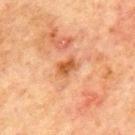Recorded during total-body skin imaging; not selected for excision or biopsy.
On the mid back.
Cropped from a total-body skin-imaging series; the visible field is about 15 mm.
This is a cross-polarized tile.
A male patient aged around 70.
The recorded lesion diameter is about 2.5 mm.
Automated image analysis of the tile measured a footprint of about 4 mm² and an eccentricity of roughly 0.75. And it measured an automated nevus-likeness rating near 35 out of 100 and a lesion-detection confidence of about 100/100.